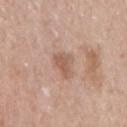The lesion was photographed on a routine skin check and not biopsied; there is no pathology result. Located on the right upper arm. The subject is a female aged approximately 60. A roughly 15 mm field-of-view crop from a total-body skin photograph.